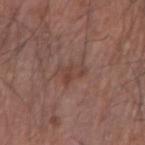Q: Is there a histopathology result?
A: imaged on a skin check; not biopsied
Q: How was this image acquired?
A: 15 mm crop, total-body photography
Q: Where on the body is the lesion?
A: the right forearm
Q: Who is the patient?
A: male, roughly 65 years of age